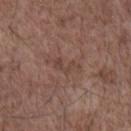* workup — no biopsy performed (imaged during a skin exam)
* diameter — ≈3 mm
* automated lesion analysis — an area of roughly 3.5 mm², a shape eccentricity near 0.85, and two-axis asymmetry of about 0.55; a border-irregularity rating of about 7/10, a color-variation rating of about 0/10, and a peripheral color-asymmetry measure near 0
* imaging modality — ~15 mm crop, total-body skin-cancer survey
* patient — male, approximately 70 years of age
* anatomic site — the chest